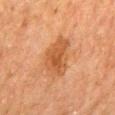The lesion is on the mid back. Longest diameter approximately 5 mm. A close-up tile cropped from a whole-body skin photograph, about 15 mm across. A male subject, approximately 80 years of age. The lesion-visualizer software estimated an average lesion color of about L≈43 a*≈21 b*≈33 (CIELAB), a lesion–skin lightness drop of about 8, and a normalized lesion–skin contrast near 7.